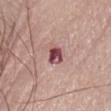{
  "biopsy_status": "not biopsied; imaged during a skin examination",
  "lesion_size": {
    "long_diameter_mm_approx": 2.5
  },
  "lighting": "white-light",
  "image": {
    "source": "total-body photography crop",
    "field_of_view_mm": 15
  },
  "patient": {
    "sex": "female",
    "age_approx": 65
  },
  "automated_metrics": {
    "area_mm2_approx": 4.5,
    "eccentricity": 0.55,
    "shape_asymmetry": 0.25
  },
  "site": "front of the torso"
}